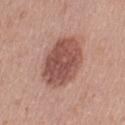Notes:
• notes · catalogued during a skin exam; not biopsied
• tile lighting · white-light
• subject · female, aged around 40
• lesion size · ≈6.5 mm
• image-analysis metrics · a lesion area of about 22 mm² and a shape eccentricity near 0.75; a border-irregularity index near 1.5/10 and radial color variation of about 1.5; a detector confidence of about 100 out of 100 that the crop contains a lesion
• image source · total-body-photography crop, ~15 mm field of view
• site · the left upper arm